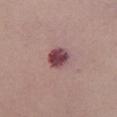Captured during whole-body skin photography for melanoma surveillance; the lesion was not biopsied. This image is a 15 mm lesion crop taken from a total-body photograph. The lesion-visualizer software estimated a lesion area of about 6 mm², an eccentricity of roughly 0.5, and a symmetry-axis asymmetry near 0.25. The analysis additionally found a border-irregularity index near 2/10 and radial color variation of about 1. And it measured an automated nevus-likeness rating near 50 out of 100 and a lesion-detection confidence of about 100/100. Longest diameter approximately 3 mm. Imaged with white-light lighting. The subject is a female in their 50s. On the chest.